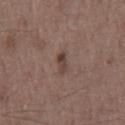Imaged during a routine full-body skin examination; the lesion was not biopsied and no histopathology is available. A male subject aged 58–62. Approximately 2.5 mm at its widest. Imaged with white-light lighting. From the chest. A region of skin cropped from a whole-body photographic capture, roughly 15 mm wide.